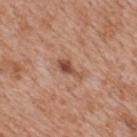• workup — catalogued during a skin exam; not biopsied
• image — ~15 mm tile from a whole-body skin photo
• lesion size — about 3.5 mm
• subject — male, aged 63 to 67
• site — the mid back
• illumination — white-light illumination